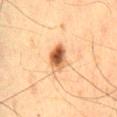Findings:
* workup — total-body-photography surveillance lesion; no biopsy
* body site — the lower back
* subject — male, aged 63 to 67
* acquisition — ~15 mm crop, total-body skin-cancer survey
* lesion diameter — ≈3.5 mm
* automated lesion analysis — an area of roughly 7 mm² and a shape-asymmetry score of about 0.25 (0 = symmetric); a lesion color around L≈51 a*≈21 b*≈35 in CIELAB